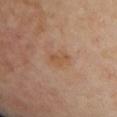notes: no biopsy performed (imaged during a skin exam) | anatomic site: the front of the torso | illumination: cross-polarized illumination | acquisition: total-body-photography crop, ~15 mm field of view | subject: female, about 40 years old | TBP lesion metrics: a border-irregularity rating of about 5/10; an automated nevus-likeness rating near 0 out of 100 | size: ~2.5 mm (longest diameter).